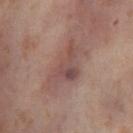lighting: cross-polarized | site: the left thigh | lesion diameter: about 5.5 mm | image: ~15 mm tile from a whole-body skin photo | patient: female, aged 53–57 | automated metrics: a lesion area of about 10 mm² and an outline eccentricity of about 0.85 (0 = round, 1 = elongated); a border-irregularity index near 6.5/10, a color-variation rating of about 5.5/10, and a peripheral color-asymmetry measure near 1.5.